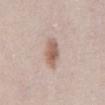Assessment: Part of a total-body skin-imaging series; this lesion was reviewed on a skin check and was not flagged for biopsy. Acquisition and patient details: Automated tile analysis of the lesion measured a footprint of about 6 mm², an eccentricity of roughly 0.8, and a shape-asymmetry score of about 0.2 (0 = symmetric). The software also gave an average lesion color of about L≈59 a*≈18 b*≈26 (CIELAB) and a normalized border contrast of about 8. The tile uses white-light illumination. A female subject aged 38–42. Located on the mid back. Cropped from a total-body skin-imaging series; the visible field is about 15 mm.